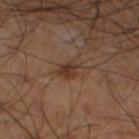Findings:
* notes — catalogued during a skin exam; not biopsied
* patient — male, aged approximately 60
* image source — ~15 mm crop, total-body skin-cancer survey
* TBP lesion metrics — border irregularity of about 3.5 on a 0–10 scale and a color-variation rating of about 3/10
* location — the left thigh
* lesion diameter — ≈3.5 mm